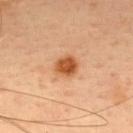workup — imaged on a skin check; not biopsied
acquisition — total-body-photography crop, ~15 mm field of view
tile lighting — cross-polarized
body site — the upper back
automated lesion analysis — a lesion area of about 6 mm², an eccentricity of roughly 0.45, and two-axis asymmetry of about 0.1; a lesion color around L≈54 a*≈27 b*≈41 in CIELAB and a lesion–skin lightness drop of about 14; peripheral color asymmetry of about 1.5; an automated nevus-likeness rating near 100 out of 100 and a detector confidence of about 100 out of 100 that the crop contains a lesion
diameter — about 2.5 mm
subject — female, approximately 35 years of age